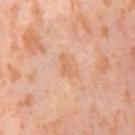{"biopsy_status": "not biopsied; imaged during a skin examination", "image": {"source": "total-body photography crop", "field_of_view_mm": 15}, "patient": {"sex": "female", "age_approx": 55}, "site": "right thigh", "automated_metrics": {"area_mm2_approx": 3.0, "eccentricity": 0.9, "shape_asymmetry": 0.5, "cielab_L": 67, "cielab_a": 23, "cielab_b": 37, "vs_skin_darker_L": 7.0, "border_irregularity_0_10": 5.0, "color_variation_0_10": 0.0, "peripheral_color_asymmetry": 0.0, "nevus_likeness_0_100": 0}, "lesion_size": {"long_diameter_mm_approx": 3.0}, "lighting": "cross-polarized"}